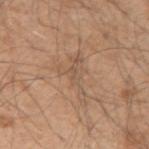<record>
  <biopsy_status>not biopsied; imaged during a skin examination</biopsy_status>
  <image>
    <source>total-body photography crop</source>
    <field_of_view_mm>15</field_of_view_mm>
  </image>
  <automated_metrics>
    <cielab_L>55</cielab_L>
    <cielab_a>17</cielab_a>
    <cielab_b>30</cielab_b>
    <vs_skin_darker_L>6.0</vs_skin_darker_L>
    <vs_skin_contrast_norm>4.5</vs_skin_contrast_norm>
    <nevus_likeness_0_100>0</nevus_likeness_0_100>
    <lesion_detection_confidence_0_100>80</lesion_detection_confidence_0_100>
  </automated_metrics>
  <lighting>white-light</lighting>
  <site>right upper arm</site>
  <patient>
    <sex>male</sex>
    <age_approx>50</age_approx>
  </patient>
  <lesion_size>
    <long_diameter_mm_approx>6.0</long_diameter_mm_approx>
  </lesion_size>
</record>